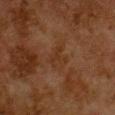Findings:
- workup · imaged on a skin check; not biopsied
- automated lesion analysis · a shape eccentricity near 0.7 and two-axis asymmetry of about 0.5; border irregularity of about 5 on a 0–10 scale, a within-lesion color-variation index near 2/10, and peripheral color asymmetry of about 0.5
- image source · 15 mm crop, total-body photography
- size · ~3.5 mm (longest diameter)
- location · the chest
- patient · female, in their 50s
- tile lighting · cross-polarized